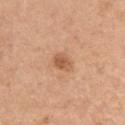Case summary:
• biopsy status — total-body-photography surveillance lesion; no biopsy
• imaging modality — 15 mm crop, total-body photography
• patient — female, aged around 65
• illumination — white-light illumination
• diameter — about 3 mm
• location — the arm
• TBP lesion metrics — an average lesion color of about L≈58 a*≈23 b*≈35 (CIELAB), about 10 CIELAB-L* units darker than the surrounding skin, and a normalized lesion–skin contrast near 7; a border-irregularity rating of about 2/10, internal color variation of about 2.5 on a 0–10 scale, and radial color variation of about 1